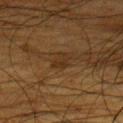biopsy status: total-body-photography surveillance lesion; no biopsy